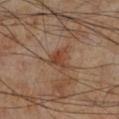Case summary:
* follow-up: catalogued during a skin exam; not biopsied